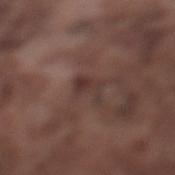Part of a total-body skin-imaging series; this lesion was reviewed on a skin check and was not flagged for biopsy.
Located on the left lower leg.
About 3.5 mm across.
Cropped from a total-body skin-imaging series; the visible field is about 15 mm.
A male subject, aged 73–77.